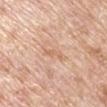{"biopsy_status": "not biopsied; imaged during a skin examination", "patient": {"sex": "male", "age_approx": 70}, "image": {"source": "total-body photography crop", "field_of_view_mm": 15}, "lighting": "white-light", "automated_metrics": {"area_mm2_approx": 2.5, "eccentricity": 0.9, "shape_asymmetry": 0.35, "cielab_L": 65, "cielab_a": 20, "cielab_b": 33, "vs_skin_darker_L": 7.0, "vs_skin_contrast_norm": 4.5}, "site": "left upper arm", "lesion_size": {"long_diameter_mm_approx": 2.5}}